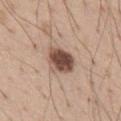Q: Is there a histopathology result?
A: catalogued during a skin exam; not biopsied
Q: What is the lesion's diameter?
A: ~3.5 mm (longest diameter)
Q: What lighting was used for the tile?
A: white-light
Q: Lesion location?
A: the chest
Q: What kind of image is this?
A: total-body-photography crop, ~15 mm field of view
Q: Automated lesion metrics?
A: an area of roughly 9.5 mm², an eccentricity of roughly 0.65, and a symmetry-axis asymmetry near 0.25; an average lesion color of about L≈49 a*≈18 b*≈25 (CIELAB) and a normalized border contrast of about 12; a classifier nevus-likeness of about 85/100 and a lesion-detection confidence of about 100/100
Q: Who is the patient?
A: male, aged 38–42